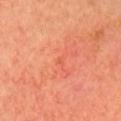The lesion was tiled from a total-body skin photograph and was not biopsied. About 1 mm across. This is a cross-polarized tile. A 15 mm close-up extracted from a 3D total-body photography capture. A female patient approximately 75 years of age. The lesion is located on the head or neck.